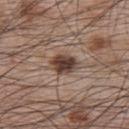| field | value |
|---|---|
| biopsy status | no biopsy performed (imaged during a skin exam) |
| lesion diameter | about 3 mm |
| lighting | white-light |
| body site | the upper back |
| patient | male, approximately 65 years of age |
| acquisition | total-body-photography crop, ~15 mm field of view |